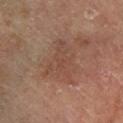<record>
<biopsy_status>not biopsied; imaged during a skin examination</biopsy_status>
<site>right leg</site>
<image>
  <source>total-body photography crop</source>
  <field_of_view_mm>15</field_of_view_mm>
</image>
<patient>
  <sex>male</sex>
  <age_approx>60</age_approx>
</patient>
<lesion_size>
  <long_diameter_mm_approx>5.5</long_diameter_mm_approx>
</lesion_size>
</record>